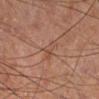Q: Was a biopsy performed?
A: no biopsy performed (imaged during a skin exam)
Q: Who is the patient?
A: male, roughly 65 years of age
Q: What is the imaging modality?
A: ~15 mm crop, total-body skin-cancer survey
Q: Where on the body is the lesion?
A: the right lower leg
Q: How was the tile lit?
A: cross-polarized illumination